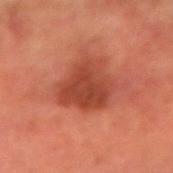lesion diameter = ≈5.5 mm | image source = total-body-photography crop, ~15 mm field of view | image-analysis metrics = an area of roughly 18 mm², a shape eccentricity near 0.45, and two-axis asymmetry of about 0.3; a border-irregularity rating of about 3/10 and a within-lesion color-variation index near 4/10; a nevus-likeness score of about 25/100 | site = the arm | subject = male, aged approximately 60 | tile lighting = cross-polarized.